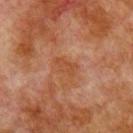Clinical impression: The lesion was photographed on a routine skin check and not biopsied; there is no pathology result. Acquisition and patient details: A male subject, aged around 80. A close-up tile cropped from a whole-body skin photograph, about 15 mm across. From the chest.